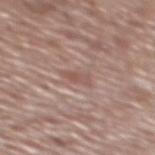notes: catalogued during a skin exam; not biopsied
tile lighting: white-light illumination
image-analysis metrics: a footprint of about 3 mm² and an outline eccentricity of about 0.85 (0 = round, 1 = elongated); an average lesion color of about L≈53 a*≈20 b*≈24 (CIELAB), roughly 7 lightness units darker than nearby skin, and a normalized border contrast of about 5.5; a classifier nevus-likeness of about 0/100 and a detector confidence of about 95 out of 100 that the crop contains a lesion
site: the mid back
image source: total-body-photography crop, ~15 mm field of view
subject: male, aged approximately 75
lesion diameter: ~2.5 mm (longest diameter)